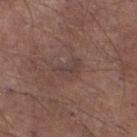Imaged during a routine full-body skin examination; the lesion was not biopsied and no histopathology is available. The subject is a male roughly 55 years of age. A 15 mm close-up extracted from a 3D total-body photography capture. The lesion is on the left lower leg.